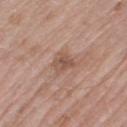Q: Was this lesion biopsied?
A: imaged on a skin check; not biopsied
Q: What lighting was used for the tile?
A: white-light
Q: How was this image acquired?
A: ~15 mm tile from a whole-body skin photo
Q: What are the patient's age and sex?
A: female, aged 68–72
Q: Where on the body is the lesion?
A: the left thigh